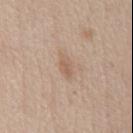Captured during whole-body skin photography for melanoma surveillance; the lesion was not biopsied.
The lesion is on the abdomen.
Cropped from a total-body skin-imaging series; the visible field is about 15 mm.
Longest diameter approximately 3 mm.
The patient is a female in their mid- to late 40s.